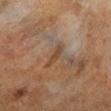<tbp_lesion>
  <lesion_size>
    <long_diameter_mm_approx>3.5</long_diameter_mm_approx>
  </lesion_size>
  <site>leg</site>
  <lighting>cross-polarized</lighting>
  <patient>
    <sex>male</sex>
    <age_approx>60</age_approx>
  </patient>
  <image>
    <source>total-body photography crop</source>
    <field_of_view_mm>15</field_of_view_mm>
  </image>
</tbp_lesion>